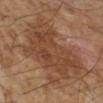A region of skin cropped from a whole-body photographic capture, roughly 15 mm wide. A male patient aged approximately 60. This is a cross-polarized tile. Located on the right lower leg.